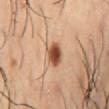The total-body-photography lesion software estimated a lesion color around L≈50 a*≈22 b*≈33 in CIELAB, roughly 17 lightness units darker than nearby skin, and a lesion-to-skin contrast of about 11.5 (normalized; higher = more distinct). It also reported a classifier nevus-likeness of about 100/100 and a lesion-detection confidence of about 100/100.
The tile uses cross-polarized illumination.
A roughly 15 mm field-of-view crop from a total-body skin photograph.
The patient is a male about 55 years old.
The lesion is located on the abdomen.